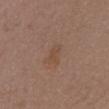Case summary:
– follow-up · no biopsy performed (imaged during a skin exam)
– patient · female, approximately 30 years of age
– body site · the head or neck
– acquisition · total-body-photography crop, ~15 mm field of view